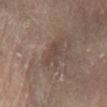The lesion was tiled from a total-body skin photograph and was not biopsied. The tile uses cross-polarized illumination. From the left lower leg. The patient is a female about 75 years old. The lesion's longest dimension is about 3.5 mm. A 15 mm close-up tile from a total-body photography series done for melanoma screening.